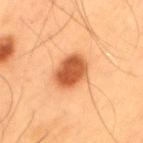<lesion>
  <biopsy_status>not biopsied; imaged during a skin examination</biopsy_status>
  <lighting>cross-polarized</lighting>
  <automated_metrics>
    <border_irregularity_0_10>1.5</border_irregularity_0_10>
    <color_variation_0_10>3.5</color_variation_0_10>
    <peripheral_color_asymmetry>1.0</peripheral_color_asymmetry>
    <nevus_likeness_0_100>100</nevus_likeness_0_100>
    <lesion_detection_confidence_0_100>100</lesion_detection_confidence_0_100>
  </automated_metrics>
  <image>
    <source>total-body photography crop</source>
    <field_of_view_mm>15</field_of_view_mm>
  </image>
  <lesion_size>
    <long_diameter_mm_approx>4.0</long_diameter_mm_approx>
  </lesion_size>
  <patient>
    <sex>male</sex>
    <age_approx>55</age_approx>
  </patient>
  <site>upper back</site>
</lesion>